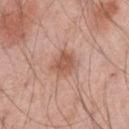A male subject, approximately 45 years of age.
The lesion is located on the left upper arm.
The tile uses white-light illumination.
A 15 mm close-up extracted from a 3D total-body photography capture.
Automated image analysis of the tile measured an average lesion color of about L≈55 a*≈23 b*≈29 (CIELAB), a lesion–skin lightness drop of about 10, and a normalized border contrast of about 7.5. The analysis additionally found a border-irregularity rating of about 2.5/10 and a within-lesion color-variation index near 2.5/10.